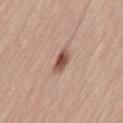Impression:
Part of a total-body skin-imaging series; this lesion was reviewed on a skin check and was not flagged for biopsy.
Acquisition and patient details:
This is a white-light tile. The lesion's longest dimension is about 3.5 mm. The lesion is located on the lower back. A 15 mm close-up tile from a total-body photography series done for melanoma screening. The lesion-visualizer software estimated a lesion–skin lightness drop of about 14 and a lesion-to-skin contrast of about 9.5 (normalized; higher = more distinct). The software also gave a border-irregularity rating of about 2.5/10, a color-variation rating of about 4.5/10, and radial color variation of about 1. A male subject, aged around 45.